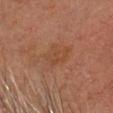Clinical impression:
Captured during whole-body skin photography for melanoma surveillance; the lesion was not biopsied.
Acquisition and patient details:
The patient is a male about 60 years old. The lesion is located on the head or neck. Imaged with cross-polarized lighting. About 3.5 mm across. This image is a 15 mm lesion crop taken from a total-body photograph. An algorithmic analysis of the crop reported an area of roughly 7 mm² and a symmetry-axis asymmetry near 0.4. The analysis additionally found an average lesion color of about L≈40 a*≈20 b*≈30 (CIELAB) and a normalized lesion–skin contrast near 5. It also reported internal color variation of about 2.5 on a 0–10 scale.